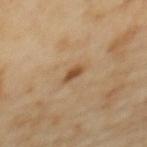This is a cross-polarized tile. The recorded lesion diameter is about 2.5 mm. A female subject, aged 58–62. Cropped from a whole-body photographic skin survey; the tile spans about 15 mm. The lesion-visualizer software estimated an eccentricity of roughly 0.9 and two-axis asymmetry of about 0.25. It also reported a lesion color around L≈50 a*≈19 b*≈36 in CIELAB and a normalized lesion–skin contrast near 8.5. The software also gave an automated nevus-likeness rating near 55 out of 100 and lesion-presence confidence of about 100/100. From the upper back.